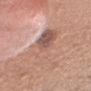• biopsy status — imaged on a skin check; not biopsied
• image source — 15 mm crop, total-body photography
• illumination — white-light
• site — the head or neck
• patient — male, about 35 years old
• lesion diameter — about 6 mm